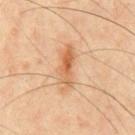This lesion was catalogued during total-body skin photography and was not selected for biopsy.
A male subject in their mid-40s.
Automated tile analysis of the lesion measured a border-irregularity rating of about 5/10.
Cropped from a whole-body photographic skin survey; the tile spans about 15 mm.
On the chest.
Longest diameter approximately 5.5 mm.